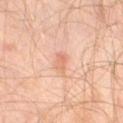This lesion was catalogued during total-body skin photography and was not selected for biopsy. A male patient aged 28–32. A lesion tile, about 15 mm wide, cut from a 3D total-body photograph. From the left leg.